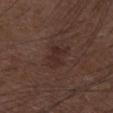lesion size = ≈3.5 mm
image = ~15 mm crop, total-body skin-cancer survey
subject = male, roughly 50 years of age
lighting = white-light
body site = the arm